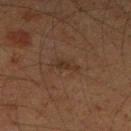The lesion is on the left thigh.
The patient is a male about 60 years old.
A region of skin cropped from a whole-body photographic capture, roughly 15 mm wide.
Automated tile analysis of the lesion measured border irregularity of about 4 on a 0–10 scale, internal color variation of about 0 on a 0–10 scale, and peripheral color asymmetry of about 0.
Captured under cross-polarized illumination.